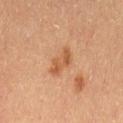Captured during whole-body skin photography for melanoma surveillance; the lesion was not biopsied. About 3.5 mm across. On the leg. A female patient, aged 58 to 62. A lesion tile, about 15 mm wide, cut from a 3D total-body photograph. The tile uses cross-polarized illumination.